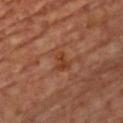anatomic site: the chest | lesion size: ~2.5 mm (longest diameter) | patient: male, aged 53 to 57 | automated metrics: a footprint of about 4.5 mm² and two-axis asymmetry of about 0.4; border irregularity of about 4 on a 0–10 scale, a color-variation rating of about 2.5/10, and a peripheral color-asymmetry measure near 1; an automated nevus-likeness rating near 0 out of 100 and lesion-presence confidence of about 100/100 | image source: 15 mm crop, total-body photography.